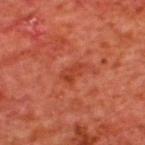Imaged during a routine full-body skin examination; the lesion was not biopsied and no histopathology is available.
A 15 mm crop from a total-body photograph taken for skin-cancer surveillance.
A male patient, in their mid- to late 60s.
From the upper back.
The tile uses cross-polarized illumination.